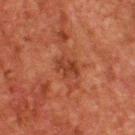The lesion was photographed on a routine skin check and not biopsied; there is no pathology result. Located on the upper back. Cropped from a whole-body photographic skin survey; the tile spans about 15 mm. A male subject, aged around 60.